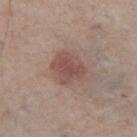Impression:
Imaged during a routine full-body skin examination; the lesion was not biopsied and no histopathology is available.
Context:
A male patient about 75 years old. A 15 mm close-up extracted from a 3D total-body photography capture. Measured at roughly 4 mm in maximum diameter. Automated image analysis of the tile measured border irregularity of about 2 on a 0–10 scale, a color-variation rating of about 3/10, and a peripheral color-asymmetry measure near 1. Located on the leg. Captured under white-light illumination.